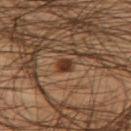On the left thigh. Cropped from a total-body skin-imaging series; the visible field is about 15 mm. A male patient, in their mid-40s.